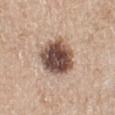  biopsy_status: not biopsied; imaged during a skin examination
  patient:
    sex: female
    age_approx: 70
  image:
    source: total-body photography crop
    field_of_view_mm: 15
  site: left lower leg
  lesion_size:
    long_diameter_mm_approx: 5.0